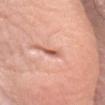The lesion was photographed on a routine skin check and not biopsied; there is no pathology result.
Measured at roughly 3 mm in maximum diameter.
Captured under white-light illumination.
A lesion tile, about 15 mm wide, cut from a 3D total-body photograph.
The subject is a female in their mid- to late 60s.
On the head or neck.